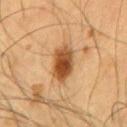Q: Was a biopsy performed?
A: total-body-photography surveillance lesion; no biopsy
Q: How was this image acquired?
A: ~15 mm crop, total-body skin-cancer survey
Q: What lighting was used for the tile?
A: cross-polarized illumination
Q: Who is the patient?
A: male, aged around 55
Q: Lesion location?
A: the chest
Q: How large is the lesion?
A: about 4.5 mm